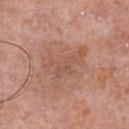Q: Is there a histopathology result?
A: no biopsy performed (imaged during a skin exam)
Q: What is the lesion's diameter?
A: ≈5.5 mm
Q: What are the patient's age and sex?
A: male, roughly 70 years of age
Q: How was the tile lit?
A: white-light illumination
Q: How was this image acquired?
A: ~15 mm crop, total-body skin-cancer survey
Q: Lesion location?
A: the front of the torso